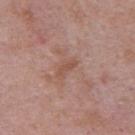notes=total-body-photography surveillance lesion; no biopsy